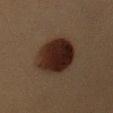Captured during whole-body skin photography for melanoma surveillance; the lesion was not biopsied. The patient is a female aged 38 to 42. Located on the left upper arm. A 15 mm close-up extracted from a 3D total-body photography capture.